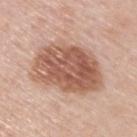Part of a total-body skin-imaging series; this lesion was reviewed on a skin check and was not flagged for biopsy. The recorded lesion diameter is about 7.5 mm. Automated tile analysis of the lesion measured a classifier nevus-likeness of about 30/100. From the upper back. This is a white-light tile. The subject is a male roughly 60 years of age. A region of skin cropped from a whole-body photographic capture, roughly 15 mm wide.